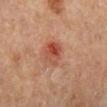Imaged during a routine full-body skin examination; the lesion was not biopsied and no histopathology is available. An algorithmic analysis of the crop reported a nevus-likeness score of about 0/100 and a detector confidence of about 100 out of 100 that the crop contains a lesion. Measured at roughly 3 mm in maximum diameter. The lesion is on the right lower leg. A 15 mm close-up extracted from a 3D total-body photography capture. A female subject about 60 years old.